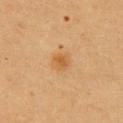  biopsy_status: not biopsied; imaged during a skin examination
  site: right upper arm
  image:
    source: total-body photography crop
    field_of_view_mm: 15
  patient:
    sex: female
    age_approx: 55